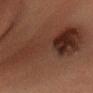workup = no biopsy performed (imaged during a skin exam)
image = ~15 mm crop, total-body skin-cancer survey
body site = the head or neck
patient = male, roughly 35 years of age